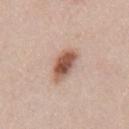The subject is a male aged 38 to 42. A 15 mm close-up extracted from a 3D total-body photography capture. Imaged with white-light lighting. The lesion is located on the back.